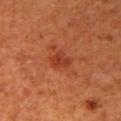This lesion was catalogued during total-body skin photography and was not selected for biopsy.
Imaged with cross-polarized lighting.
The subject is a female roughly 50 years of age.
On the right upper arm.
A lesion tile, about 15 mm wide, cut from a 3D total-body photograph.
An algorithmic analysis of the crop reported a lesion area of about 4 mm², an eccentricity of roughly 0.65, and a shape-asymmetry score of about 0.3 (0 = symmetric). And it measured a border-irregularity index near 2.5/10, internal color variation of about 2.5 on a 0–10 scale, and peripheral color asymmetry of about 1. The software also gave lesion-presence confidence of about 100/100.